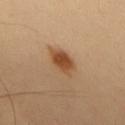lesion_size:
  long_diameter_mm_approx: 4.0
lighting: cross-polarized
image:
  source: total-body photography crop
  field_of_view_mm: 15
automated_metrics:
  area_mm2_approx: 7.5
  shape_asymmetry: 0.2
  nevus_likeness_0_100: 95
  lesion_detection_confidence_0_100: 100
site: upper back
patient:
  sex: male
  age_approx: 45